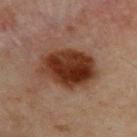A male subject in their mid- to late 40s. The lesion is located on the upper back. Cropped from a whole-body photographic skin survey; the tile spans about 15 mm. Automated image analysis of the tile measured a lesion color around L≈29 a*≈20 b*≈26 in CIELAB, a lesion–skin lightness drop of about 14, and a normalized lesion–skin contrast near 13.5. And it measured a border-irregularity rating of about 2/10, a within-lesion color-variation index near 6.5/10, and radial color variation of about 2.5. The software also gave a nevus-likeness score of about 100/100 and lesion-presence confidence of about 100/100. Measured at roughly 6.5 mm in maximum diameter. Captured under cross-polarized illumination.